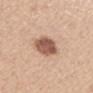The lesion was photographed on a routine skin check and not biopsied; there is no pathology result. A close-up tile cropped from a whole-body skin photograph, about 15 mm across. Captured under white-light illumination. Located on the mid back. A female patient, about 50 years old. Measured at roughly 4 mm in maximum diameter.